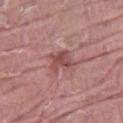lighting=white-light | site=the right thigh | image source=total-body-photography crop, ~15 mm field of view | patient=male, aged 38–42.